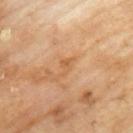  biopsy_status: not biopsied; imaged during a skin examination
  site: back
  lesion_size:
    long_diameter_mm_approx: 2.5
  patient:
    sex: male
    age_approx: 70
  image:
    source: total-body photography crop
    field_of_view_mm: 15
  automated_metrics:
    cielab_L: 58
    cielab_a: 22
    cielab_b: 39
    vs_skin_darker_L: 6.0
    vs_skin_contrast_norm: 5.0
  lighting: cross-polarized